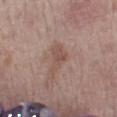About 4 mm across.
The lesion is located on the right forearm.
Automated image analysis of the tile measured an area of roughly 5 mm², an outline eccentricity of about 0.85 (0 = round, 1 = elongated), and two-axis asymmetry of about 0.55. And it measured a border-irregularity rating of about 5.5/10, a color-variation rating of about 2/10, and peripheral color asymmetry of about 0.5.
A roughly 15 mm field-of-view crop from a total-body skin photograph.
The tile uses white-light illumination.
A female patient, aged around 70.